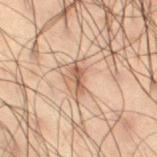• follow-up — no biopsy performed (imaged during a skin exam)
• anatomic site — the right thigh
• imaging modality — total-body-photography crop, ~15 mm field of view
• image-analysis metrics — an area of roughly 3 mm², an outline eccentricity of about 0.9 (0 = round, 1 = elongated), and a shape-asymmetry score of about 0.35 (0 = symmetric); internal color variation of about 1 on a 0–10 scale and peripheral color asymmetry of about 0
• illumination — cross-polarized illumination
• patient — male, aged around 50